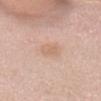This lesion was catalogued during total-body skin photography and was not selected for biopsy. From the head or neck. Imaged with white-light lighting. The subject is a female aged approximately 20. This image is a 15 mm lesion crop taken from a total-body photograph. Longest diameter approximately 3 mm.